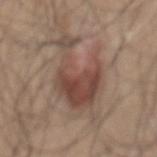anatomic site — the mid back
acquisition — 15 mm crop, total-body photography
patient — male, roughly 35 years of age